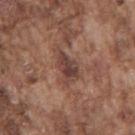Notes:
– notes: total-body-photography surveillance lesion; no biopsy
– acquisition: total-body-photography crop, ~15 mm field of view
– patient: male, in their mid- to late 70s
– anatomic site: the back